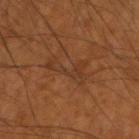Impression: This lesion was catalogued during total-body skin photography and was not selected for biopsy. Acquisition and patient details: From the right forearm. The lesion's longest dimension is about 5 mm. Cropped from a whole-body photographic skin survey; the tile spans about 15 mm. The subject is a male aged around 55. Automated tile analysis of the lesion measured lesion-presence confidence of about 95/100. The tile uses cross-polarized illumination.